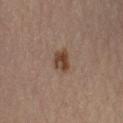Imaged during a routine full-body skin examination; the lesion was not biopsied and no histopathology is available. The lesion's longest dimension is about 2.5 mm. On the left upper arm. A male subject in their mid-50s. A close-up tile cropped from a whole-body skin photograph, about 15 mm across. Imaged with cross-polarized lighting.